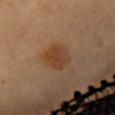| field | value |
|---|---|
| biopsy status | catalogued during a skin exam; not biopsied |
| image | ~15 mm crop, total-body skin-cancer survey |
| site | the chest |
| patient | female, in their mid- to late 60s |